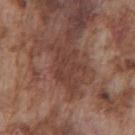No biopsy was performed on this lesion — it was imaged during a full skin examination and was not determined to be concerning. A male patient roughly 75 years of age. Automated image analysis of the tile measured a classifier nevus-likeness of about 0/100 and a detector confidence of about 85 out of 100 that the crop contains a lesion. From the chest. A 15 mm close-up tile from a total-body photography series done for melanoma screening. Approximately 5 mm at its widest. Captured under white-light illumination.